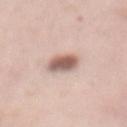Recorded during total-body skin imaging; not selected for excision or biopsy.
Automated tile analysis of the lesion measured a border-irregularity rating of about 1.5/10, a within-lesion color-variation index near 4.5/10, and radial color variation of about 1. And it measured a nevus-likeness score of about 85/100 and lesion-presence confidence of about 100/100.
Approximately 3 mm at its widest.
A female patient in their mid-30s.
Cropped from a whole-body photographic skin survey; the tile spans about 15 mm.
Imaged with white-light lighting.
The lesion is on the chest.